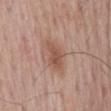subject — male, aged 63–67; illumination — white-light illumination; site — the mid back; size — ≈4.5 mm; acquisition — ~15 mm tile from a whole-body skin photo.